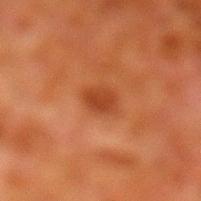The lesion was photographed on a routine skin check and not biopsied; there is no pathology result. Imaged with cross-polarized lighting. On the leg. The lesion's longest dimension is about 2.5 mm. This image is a 15 mm lesion crop taken from a total-body photograph. A male subject aged approximately 80.